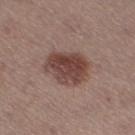Findings:
• notes: catalogued during a skin exam; not biopsied
• illumination: white-light
• TBP lesion metrics: a symmetry-axis asymmetry near 0.2; border irregularity of about 2 on a 0–10 scale and internal color variation of about 4.5 on a 0–10 scale
• subject: female, aged around 40
• lesion diameter: ~5 mm (longest diameter)
• image: ~15 mm crop, total-body skin-cancer survey
• body site: the right thigh